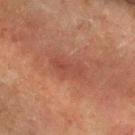Captured during whole-body skin photography for melanoma surveillance; the lesion was not biopsied.
Approximately 4 mm at its widest.
This image is a 15 mm lesion crop taken from a total-body photograph.
Located on the front of the torso.
The subject is a female in their mid-50s.
Captured under cross-polarized illumination.
The total-body-photography lesion software estimated an area of roughly 5.5 mm² and two-axis asymmetry of about 0.4. The analysis additionally found a normalized border contrast of about 5. It also reported border irregularity of about 5 on a 0–10 scale and a peripheral color-asymmetry measure near 0.5. It also reported an automated nevus-likeness rating near 0 out of 100 and a lesion-detection confidence of about 100/100.